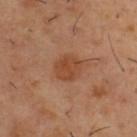This lesion was catalogued during total-body skin photography and was not selected for biopsy. A close-up tile cropped from a whole-body skin photograph, about 15 mm across. Located on the upper back. Automated tile analysis of the lesion measured an area of roughly 7.5 mm², an eccentricity of roughly 0.5, and two-axis asymmetry of about 0.15. The analysis additionally found an average lesion color of about L≈44 a*≈23 b*≈33 (CIELAB) and a lesion–skin lightness drop of about 8. A male patient approximately 55 years of age. The tile uses cross-polarized illumination.